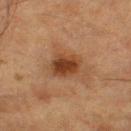<record>
  <biopsy_status>not biopsied; imaged during a skin examination</biopsy_status>
  <image>
    <source>total-body photography crop</source>
    <field_of_view_mm>15</field_of_view_mm>
  </image>
  <site>leg</site>
  <patient>
    <sex>male</sex>
    <age_approx>85+</age_approx>
  </patient>
</record>